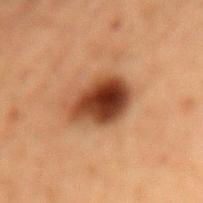The lesion was tiled from a total-body skin photograph and was not biopsied.